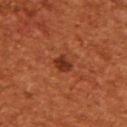This lesion was catalogued during total-body skin photography and was not selected for biopsy.
Automated image analysis of the tile measured a mean CIELAB color near L≈32 a*≈28 b*≈32 and a normalized border contrast of about 9. And it measured border irregularity of about 2 on a 0–10 scale.
The patient is a male in their mid-40s.
Cropped from a whole-body photographic skin survey; the tile spans about 15 mm.
This is a cross-polarized tile.
From the upper back.